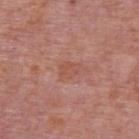<tbp_lesion>
  <biopsy_status>not biopsied; imaged during a skin examination</biopsy_status>
  <lighting>white-light</lighting>
  <patient>
    <sex>male</sex>
    <age_approx>75</age_approx>
  </patient>
  <automated_metrics>
    <area_mm2_approx>4.0</area_mm2_approx>
    <eccentricity>0.75</eccentricity>
    <shape_asymmetry>0.3</shape_asymmetry>
  </automated_metrics>
  <lesion_size>
    <long_diameter_mm_approx>2.5</long_diameter_mm_approx>
  </lesion_size>
  <site>upper back</site>
  <image>
    <source>total-body photography crop</source>
    <field_of_view_mm>15</field_of_view_mm>
  </image>
</tbp_lesion>